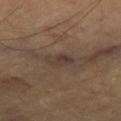Q: Is there a histopathology result?
A: no biopsy performed (imaged during a skin exam)
Q: Where on the body is the lesion?
A: the right thigh
Q: What is the lesion's diameter?
A: ~3.5 mm (longest diameter)
Q: What is the imaging modality?
A: 15 mm crop, total-body photography
Q: What are the patient's age and sex?
A: about 65 years old